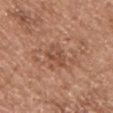The lesion was photographed on a routine skin check and not biopsied; there is no pathology result. The patient is a male about 75 years old. A 15 mm close-up tile from a total-body photography series done for melanoma screening. From the chest. The tile uses white-light illumination. Longest diameter approximately 4 mm. The lesion-visualizer software estimated a lesion color around L≈50 a*≈22 b*≈31 in CIELAB, about 8 CIELAB-L* units darker than the surrounding skin, and a lesion-to-skin contrast of about 6 (normalized; higher = more distinct).